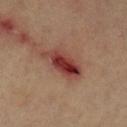Impression:
Recorded during total-body skin imaging; not selected for excision or biopsy.
Context:
A roughly 15 mm field-of-view crop from a total-body skin photograph. About 5 mm across. The subject is aged 58 to 62. From the left lower leg.